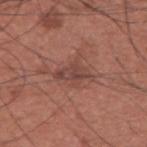No biopsy was performed on this lesion — it was imaged during a full skin examination and was not determined to be concerning. The lesion is on the upper back. The patient is a male aged approximately 35. The total-body-photography lesion software estimated a lesion area of about 6 mm², an eccentricity of roughly 0.85, and two-axis asymmetry of about 0.3. And it measured an automated nevus-likeness rating near 0 out of 100 and a lesion-detection confidence of about 95/100. Imaged with white-light lighting. A 15 mm crop from a total-body photograph taken for skin-cancer surveillance.